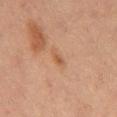The lesion was tiled from a total-body skin photograph and was not biopsied. Measured at roughly 2.5 mm in maximum diameter. A close-up tile cropped from a whole-body skin photograph, about 15 mm across. This is a cross-polarized tile. A male patient, in their mid-60s. On the abdomen.